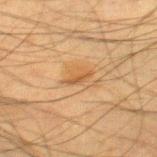{"biopsy_status": "not biopsied; imaged during a skin examination", "image": {"source": "total-body photography crop", "field_of_view_mm": 15}, "site": "right thigh", "patient": {"sex": "male", "age_approx": 35}, "lighting": "cross-polarized", "lesion_size": {"long_diameter_mm_approx": 3.0}}